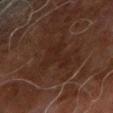{"biopsy_status": "not biopsied; imaged during a skin examination", "lighting": "cross-polarized", "image": {"source": "total-body photography crop", "field_of_view_mm": 15}, "site": "right forearm", "patient": {"sex": "male", "age_approx": 70}}